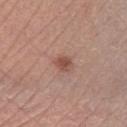<case>
<site>left lower leg</site>
<image>
  <source>total-body photography crop</source>
  <field_of_view_mm>15</field_of_view_mm>
</image>
<lesion_size>
  <long_diameter_mm_approx>2.0</long_diameter_mm_approx>
</lesion_size>
<patient>
  <sex>male</sex>
  <age_approx>65</age_approx>
</patient>
<lighting>white-light</lighting>
<automated_metrics>
  <cielab_L>50</cielab_L>
  <cielab_a>22</cielab_a>
  <cielab_b>27</cielab_b>
  <vs_skin_contrast_norm>7.5</vs_skin_contrast_norm>
  <nevus_likeness_0_100>65</nevus_likeness_0_100>
  <lesion_detection_confidence_0_100>100</lesion_detection_confidence_0_100>
</automated_metrics>
</case>